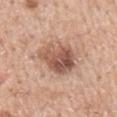Assessment:
Captured during whole-body skin photography for melanoma surveillance; the lesion was not biopsied.
Clinical summary:
The subject is a male aged 58–62. On the right upper arm. Cropped from a whole-body photographic skin survey; the tile spans about 15 mm.